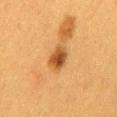{"biopsy_status": "not biopsied; imaged during a skin examination", "image": {"source": "total-body photography crop", "field_of_view_mm": 15}, "patient": {"sex": "female", "age_approx": 40}, "site": "mid back"}